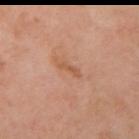<lesion>
<biopsy_status>not biopsied; imaged during a skin examination</biopsy_status>
<patient>
  <sex>female</sex>
  <age_approx>55</age_approx>
</patient>
<site>right upper arm</site>
<image>
  <source>total-body photography crop</source>
  <field_of_view_mm>15</field_of_view_mm>
</image>
<lighting>cross-polarized</lighting>
<lesion_size>
  <long_diameter_mm_approx>3.0</long_diameter_mm_approx>
</lesion_size>
</lesion>